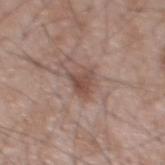follow-up — catalogued during a skin exam; not biopsied | site — the chest | image source — ~15 mm tile from a whole-body skin photo | subject — male, aged around 65.